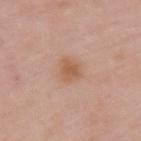{
  "lighting": "white-light",
  "site": "upper back",
  "image": {
    "source": "total-body photography crop",
    "field_of_view_mm": 15
  },
  "patient": {
    "sex": "male",
    "age_approx": 50
  },
  "automated_metrics": {
    "cielab_L": 57,
    "cielab_a": 21,
    "cielab_b": 33,
    "border_irregularity_0_10": 2.5,
    "color_variation_0_10": 2.0,
    "peripheral_color_asymmetry": 0.5,
    "nevus_likeness_0_100": 85,
    "lesion_detection_confidence_0_100": 100
  },
  "lesion_size": {
    "long_diameter_mm_approx": 2.5
  }
}